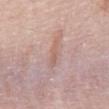Notes:
* biopsy status — catalogued during a skin exam; not biopsied
* image source — total-body-photography crop, ~15 mm field of view
* site — the abdomen
* lighting — white-light
* subject — male, aged around 70